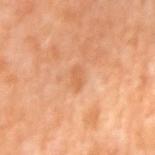<tbp_lesion>
  <biopsy_status>not biopsied; imaged during a skin examination</biopsy_status>
  <patient>
    <sex>female</sex>
    <age_approx>50</age_approx>
  </patient>
  <image>
    <source>total-body photography crop</source>
    <field_of_view_mm>15</field_of_view_mm>
  </image>
  <lighting>cross-polarized</lighting>
  <lesion_size>
    <long_diameter_mm_approx>2.5</long_diameter_mm_approx>
  </lesion_size>
  <site>left upper arm</site>
</tbp_lesion>